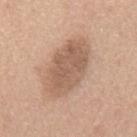The lesion was tiled from a total-body skin photograph and was not biopsied. Approximately 7.5 mm at its widest. A 15 mm close-up extracted from a 3D total-body photography capture. A male subject aged around 60. The lesion is located on the mid back.